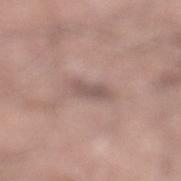This lesion was catalogued during total-body skin photography and was not selected for biopsy. The lesion is on the right lower leg. This image is a 15 mm lesion crop taken from a total-body photograph. A male patient in their mid-40s.